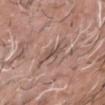notes: no biopsy performed (imaged during a skin exam) | location: the head or neck | lesion diameter: about 4.5 mm | illumination: white-light illumination | automated metrics: a within-lesion color-variation index near 4/10 and radial color variation of about 1 | imaging modality: ~15 mm crop, total-body skin-cancer survey | subject: male, approximately 70 years of age.